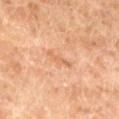Clinical impression:
Captured during whole-body skin photography for melanoma surveillance; the lesion was not biopsied.
Image and clinical context:
The tile uses cross-polarized illumination. Located on the left lower leg. A female patient, in their 70s. A close-up tile cropped from a whole-body skin photograph, about 15 mm across. The lesion's longest dimension is about 3 mm.